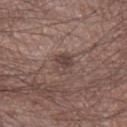biopsy status = imaged on a skin check; not biopsied
patient = male, aged 63–67
location = the right lower leg
imaging modality = ~15 mm tile from a whole-body skin photo
tile lighting = white-light illumination
size = ~2.5 mm (longest diameter)